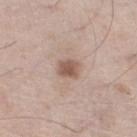Clinical impression:
Part of a total-body skin-imaging series; this lesion was reviewed on a skin check and was not flagged for biopsy.
Acquisition and patient details:
Imaged with white-light lighting. A 15 mm close-up tile from a total-body photography series done for melanoma screening. A male patient approximately 60 years of age. The lesion's longest dimension is about 2.5 mm. The lesion is located on the left lower leg. An algorithmic analysis of the crop reported an area of roughly 4 mm², an outline eccentricity of about 0.55 (0 = round, 1 = elongated), and a symmetry-axis asymmetry near 0.25. It also reported an average lesion color of about L≈55 a*≈18 b*≈26 (CIELAB), a lesion–skin lightness drop of about 12, and a lesion-to-skin contrast of about 8.5 (normalized; higher = more distinct). The software also gave a classifier nevus-likeness of about 70/100.